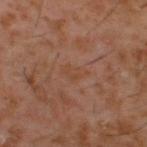follow-up: imaged on a skin check; not biopsied | location: the upper back | patient: male, aged 58–62 | imaging modality: total-body-photography crop, ~15 mm field of view.